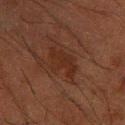This lesion was catalogued during total-body skin photography and was not selected for biopsy. The lesion is located on the right forearm. A region of skin cropped from a whole-body photographic capture, roughly 15 mm wide. The patient is a male in their 60s.